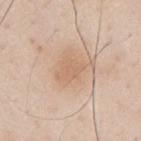Findings:
* workup: imaged on a skin check; not biopsied
* image: ~15 mm tile from a whole-body skin photo
* body site: the left upper arm
* subject: male, aged around 40
* lighting: white-light illumination